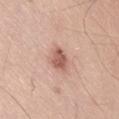biopsy status: imaged on a skin check; not biopsied | automated metrics: a mean CIELAB color near L≈57 a*≈24 b*≈27, about 13 CIELAB-L* units darker than the surrounding skin, and a lesion-to-skin contrast of about 8.5 (normalized; higher = more distinct); border irregularity of about 2.5 on a 0–10 scale, internal color variation of about 2.5 on a 0–10 scale, and a peripheral color-asymmetry measure near 1; a classifier nevus-likeness of about 85/100 and a detector confidence of about 100 out of 100 that the crop contains a lesion | subject: male, roughly 40 years of age | tile lighting: white-light illumination | body site: the right thigh | acquisition: ~15 mm tile from a whole-body skin photo | size: about 2.5 mm.